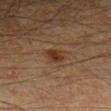Clinical impression: The lesion was photographed on a routine skin check and not biopsied; there is no pathology result. Clinical summary: The lesion is located on the arm. Measured at roughly 2.5 mm in maximum diameter. A close-up tile cropped from a whole-body skin photograph, about 15 mm across. Automated tile analysis of the lesion measured an area of roughly 4.5 mm², a shape eccentricity near 0.65, and a shape-asymmetry score of about 0.15 (0 = symmetric). It also reported a lesion color around L≈27 a*≈15 b*≈25 in CIELAB, a lesion–skin lightness drop of about 7, and a lesion-to-skin contrast of about 8.5 (normalized; higher = more distinct). And it measured a classifier nevus-likeness of about 75/100 and a detector confidence of about 100 out of 100 that the crop contains a lesion. This is a cross-polarized tile. The subject is a male in their 50s.